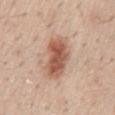location — the mid back
diameter — ~5 mm (longest diameter)
imaging modality — 15 mm crop, total-body photography
automated lesion analysis — an area of roughly 15 mm², an outline eccentricity of about 0.8 (0 = round, 1 = elongated), and a symmetry-axis asymmetry near 0.2; a mean CIELAB color near L≈57 a*≈22 b*≈30; a border-irregularity rating of about 2/10, a within-lesion color-variation index near 6/10, and peripheral color asymmetry of about 2
patient — male, in their mid-50s
tile lighting — white-light illumination